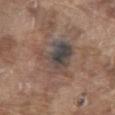  biopsy_status: not biopsied; imaged during a skin examination
  lighting: white-light
  image:
    source: total-body photography crop
    field_of_view_mm: 15
  patient:
    sex: male
    age_approx: 80
  site: chest